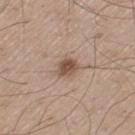follow-up: total-body-photography surveillance lesion; no biopsy
patient: male, aged 58 to 62
image source: 15 mm crop, total-body photography
size: ≈3 mm
lighting: white-light
site: the left thigh